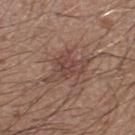Clinical impression:
Captured during whole-body skin photography for melanoma surveillance; the lesion was not biopsied.
Context:
A male subject aged approximately 20. Automated tile analysis of the lesion measured an average lesion color of about L≈44 a*≈19 b*≈23 (CIELAB), roughly 8 lightness units darker than nearby skin, and a normalized border contrast of about 6.5. The analysis additionally found a within-lesion color-variation index near 3.5/10 and peripheral color asymmetry of about 1. This is a white-light tile. A 15 mm close-up tile from a total-body photography series done for melanoma screening. The lesion's longest dimension is about 5.5 mm. The lesion is on the leg.